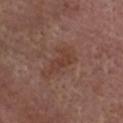Imaged during a routine full-body skin examination; the lesion was not biopsied and no histopathology is available.